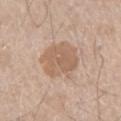automated metrics = a nevus-likeness score of about 10/100
site = the right thigh
lighting = white-light
acquisition = 15 mm crop, total-body photography
lesion diameter = ≈5.5 mm
patient = male, aged 63 to 67